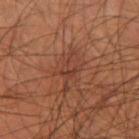Clinical impression: Part of a total-body skin-imaging series; this lesion was reviewed on a skin check and was not flagged for biopsy. Acquisition and patient details: A male patient, in their mid- to late 50s. Located on the left thigh. Measured at roughly 5 mm in maximum diameter. A region of skin cropped from a whole-body photographic capture, roughly 15 mm wide. Imaged with cross-polarized lighting. An algorithmic analysis of the crop reported a lesion color around L≈36 a*≈22 b*≈27 in CIELAB, roughly 6 lightness units darker than nearby skin, and a lesion-to-skin contrast of about 5.5 (normalized; higher = more distinct).